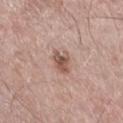Acquisition and patient details:
A 15 mm crop from a total-body photograph taken for skin-cancer surveillance. The patient is a male aged approximately 75. The lesion-visualizer software estimated an area of roughly 5 mm², a shape eccentricity near 0.8, and two-axis asymmetry of about 0.3. It also reported a mean CIELAB color near L≈54 a*≈20 b*≈25 and a normalized border contrast of about 8. And it measured a border-irregularity rating of about 3.5/10, a color-variation rating of about 4/10, and peripheral color asymmetry of about 1. And it measured a nevus-likeness score of about 75/100. Approximately 3 mm at its widest. From the right thigh.